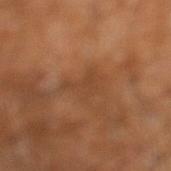workup = imaged on a skin check; not biopsied
patient = male, aged approximately 60
diameter = ~4.5 mm (longest diameter)
location = the right leg
automated metrics = a lesion color around L≈41 a*≈21 b*≈32 in CIELAB, about 6 CIELAB-L* units darker than the surrounding skin, and a normalized lesion–skin contrast near 5; a classifier nevus-likeness of about 0/100
imaging modality = ~15 mm crop, total-body skin-cancer survey